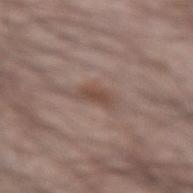| key | value |
|---|---|
| biopsy status | total-body-photography surveillance lesion; no biopsy |
| tile lighting | white-light illumination |
| automated lesion analysis | an area of roughly 3 mm²; a color-variation rating of about 0.5/10 and peripheral color asymmetry of about 0; an automated nevus-likeness rating near 50 out of 100 |
| location | the arm |
| diameter | about 3 mm |
| image source | 15 mm crop, total-body photography |
| subject | male, aged 68 to 72 |